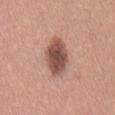biopsy status=total-body-photography surveillance lesion; no biopsy
site=the abdomen
diameter=about 6 mm
acquisition=total-body-photography crop, ~15 mm field of view
lighting=white-light
TBP lesion metrics=a lesion color around L≈51 a*≈23 b*≈25 in CIELAB, a lesion–skin lightness drop of about 15, and a normalized lesion–skin contrast near 10; a border-irregularity rating of about 2/10 and a peripheral color-asymmetry measure near 1.5; a nevus-likeness score of about 95/100 and a lesion-detection confidence of about 100/100
patient=male, aged around 35